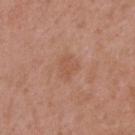Case summary:
• anatomic site · the upper back
• acquisition · total-body-photography crop, ~15 mm field of view
• patient · female, aged approximately 40